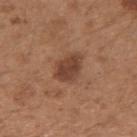Assessment: Recorded during total-body skin imaging; not selected for excision or biopsy. Background: The lesion's longest dimension is about 4 mm. Automated image analysis of the tile measured a border-irregularity index near 2/10, a within-lesion color-variation index near 2.5/10, and peripheral color asymmetry of about 1. The software also gave a classifier nevus-likeness of about 65/100 and a detector confidence of about 100 out of 100 that the crop contains a lesion. A female patient about 30 years old. Located on the left forearm. Cropped from a total-body skin-imaging series; the visible field is about 15 mm.